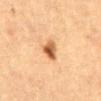{"patient": {"sex": "female", "age_approx": 60}, "site": "abdomen", "lighting": "cross-polarized", "automated_metrics": {"area_mm2_approx": 5.0, "eccentricity": 0.7, "shape_asymmetry": 0.3, "cielab_L": 48, "cielab_a": 20, "cielab_b": 34, "vs_skin_darker_L": 14.0, "vs_skin_contrast_norm": 10.0, "nevus_likeness_0_100": 95, "lesion_detection_confidence_0_100": 100}, "lesion_size": {"long_diameter_mm_approx": 3.0}, "image": {"source": "total-body photography crop", "field_of_view_mm": 15}}